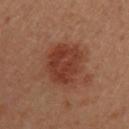biopsy status=imaged on a skin check; not biopsied
patient=female, aged 28–32
location=the left upper arm
acquisition=~15 mm tile from a whole-body skin photo
lighting=cross-polarized